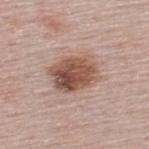Q: Was this lesion biopsied?
A: imaged on a skin check; not biopsied
Q: Patient demographics?
A: male, roughly 60 years of age
Q: Where on the body is the lesion?
A: the upper back
Q: How was this image acquired?
A: ~15 mm crop, total-body skin-cancer survey